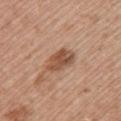biopsy status: no biopsy performed (imaged during a skin exam)
image: 15 mm crop, total-body photography
lighting: white-light illumination
body site: the upper back
lesion diameter: ~4.5 mm (longest diameter)
automated lesion analysis: a lesion color around L≈52 a*≈21 b*≈31 in CIELAB, roughly 12 lightness units darker than nearby skin, and a normalized border contrast of about 8.5; a classifier nevus-likeness of about 40/100 and a lesion-detection confidence of about 100/100
subject: female, about 60 years old